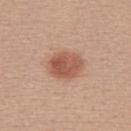Case summary:
- workup — total-body-photography surveillance lesion; no biopsy
- image-analysis metrics — a within-lesion color-variation index near 4/10
- acquisition — 15 mm crop, total-body photography
- tile lighting — white-light
- body site — the back
- subject — female, approximately 40 years of age
- size — about 4.5 mm